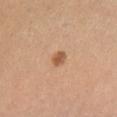<lesion>
  <biopsy_status>not biopsied; imaged during a skin examination</biopsy_status>
  <site>right lower leg</site>
  <automated_metrics>
    <vs_skin_darker_L>11.0</vs_skin_darker_L>
    <vs_skin_contrast_norm>8.0</vs_skin_contrast_norm>
    <lesion_detection_confidence_0_100>100</lesion_detection_confidence_0_100>
  </automated_metrics>
  <image>
    <source>total-body photography crop</source>
    <field_of_view_mm>15</field_of_view_mm>
  </image>
  <patient>
    <sex>female</sex>
    <age_approx>55</age_approx>
  </patient>
  <lesion_size>
    <long_diameter_mm_approx>2.0</long_diameter_mm_approx>
  </lesion_size>
  <lighting>cross-polarized</lighting>
</lesion>